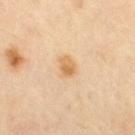TBP lesion metrics: a footprint of about 4 mm² and two-axis asymmetry of about 0.25; border irregularity of about 2 on a 0–10 scale, a color-variation rating of about 4/10, and peripheral color asymmetry of about 1.5; an automated nevus-likeness rating near 95 out of 100 and lesion-presence confidence of about 100/100 | tile lighting: cross-polarized illumination | subject: male, approximately 65 years of age | size: about 2.5 mm | imaging modality: ~15 mm crop, total-body skin-cancer survey | location: the right thigh.